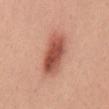Impression:
No biopsy was performed on this lesion — it was imaged during a full skin examination and was not determined to be concerning.
Image and clinical context:
Captured under white-light illumination. Automated image analysis of the tile measured a border-irregularity rating of about 1.5/10, a color-variation rating of about 6.5/10, and peripheral color asymmetry of about 2. The analysis additionally found a classifier nevus-likeness of about 100/100 and a lesion-detection confidence of about 100/100. A female subject aged around 40. A 15 mm crop from a total-body photograph taken for skin-cancer surveillance. From the chest. About 6 mm across.